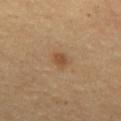Q: Is there a histopathology result?
A: total-body-photography surveillance lesion; no biopsy
Q: What are the patient's age and sex?
A: male, roughly 60 years of age
Q: How was this image acquired?
A: total-body-photography crop, ~15 mm field of view
Q: What is the lesion's diameter?
A: ~2.5 mm (longest diameter)
Q: What is the anatomic site?
A: the chest